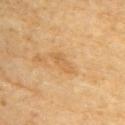  patient:
    sex: female
    age_approx: 70
  image:
    source: total-body photography crop
    field_of_view_mm: 15
  automated_metrics:
    cielab_L: 64
    cielab_a: 21
    cielab_b: 45
    vs_skin_contrast_norm: 5.5
    border_irregularity_0_10: 3.5
    color_variation_0_10: 1.0
    peripheral_color_asymmetry: 0.0
  lighting: cross-polarized
  site: right upper arm